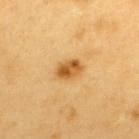Impression: This lesion was catalogued during total-body skin photography and was not selected for biopsy. Image and clinical context: A 15 mm close-up extracted from a 3D total-body photography capture. The recorded lesion diameter is about 3.5 mm. A male patient, aged 58–62. The lesion is on the back. An algorithmic analysis of the crop reported a mean CIELAB color near L≈58 a*≈22 b*≈47, a lesion–skin lightness drop of about 13, and a normalized lesion–skin contrast near 9. It also reported a border-irregularity index near 2/10, a within-lesion color-variation index near 5.5/10, and peripheral color asymmetry of about 2. And it measured a nevus-likeness score of about 95/100 and a detector confidence of about 100 out of 100 that the crop contains a lesion. This is a cross-polarized tile.